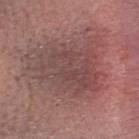Assessment: Imaged during a routine full-body skin examination; the lesion was not biopsied and no histopathology is available. Image and clinical context: The total-body-photography lesion software estimated a mean CIELAB color near L≈45 a*≈21 b*≈20 and a lesion-to-skin contrast of about 5.5 (normalized; higher = more distinct). The analysis additionally found an automated nevus-likeness rating near 0 out of 100. A 15 mm close-up extracted from a 3D total-body photography capture. Imaged with white-light lighting. Longest diameter approximately 7.5 mm. On the head or neck. A female patient aged 38 to 42.